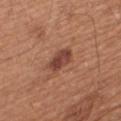Clinical impression: This lesion was catalogued during total-body skin photography and was not selected for biopsy. Image and clinical context: A male patient, aged approximately 65. A 15 mm close-up tile from a total-body photography series done for melanoma screening. Longest diameter approximately 3.5 mm. Located on the upper back.